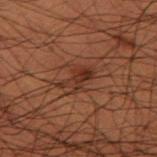biopsy_status: not biopsied; imaged during a skin examination
site: left thigh
image:
  source: total-body photography crop
  field_of_view_mm: 15
lesion_size:
  long_diameter_mm_approx: 4.0
patient:
  sex: male
  age_approx: 50